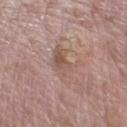Clinical impression:
Part of a total-body skin-imaging series; this lesion was reviewed on a skin check and was not flagged for biopsy.
Acquisition and patient details:
This is a white-light tile. A female subject aged around 70. The lesion is on the right lower leg. A 15 mm close-up extracted from a 3D total-body photography capture. The recorded lesion diameter is about 5 mm.